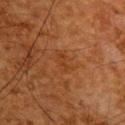<record>
<biopsy_status>not biopsied; imaged during a skin examination</biopsy_status>
<patient>
  <sex>male</sex>
  <age_approx>65</age_approx>
</patient>
<lighting>cross-polarized</lighting>
<lesion_size>
  <long_diameter_mm_approx>3.0</long_diameter_mm_approx>
</lesion_size>
<site>upper back</site>
<image>
  <source>total-body photography crop</source>
  <field_of_view_mm>15</field_of_view_mm>
</image>
</record>